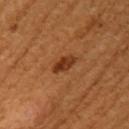Imaged during a routine full-body skin examination; the lesion was not biopsied and no histopathology is available. The lesion is on the left upper arm. The recorded lesion diameter is about 3 mm. A 15 mm close-up tile from a total-body photography series done for melanoma screening. The patient is a female aged 53 to 57. Imaged with cross-polarized lighting.